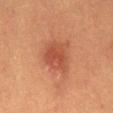Findings:
– notes · total-body-photography surveillance lesion; no biopsy
– body site · the mid back
– image source · ~15 mm crop, total-body skin-cancer survey
– size · about 4 mm
– patient · female, in their mid- to late 50s